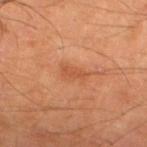biopsy_status: not biopsied; imaged during a skin examination
site: right lower leg
lesion_size:
  long_diameter_mm_approx: 3.0
patient:
  sex: male
  age_approx: 65
lighting: cross-polarized
image:
  source: total-body photography crop
  field_of_view_mm: 15